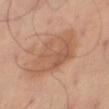Case summary:
- workup: total-body-photography surveillance lesion; no biopsy
- patient: male, aged approximately 45
- site: the right thigh
- image: 15 mm crop, total-body photography